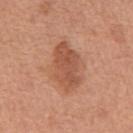Impression:
Imaged during a routine full-body skin examination; the lesion was not biopsied and no histopathology is available.
Acquisition and patient details:
The recorded lesion diameter is about 6 mm. A male patient, roughly 65 years of age. A lesion tile, about 15 mm wide, cut from a 3D total-body photograph. Automated tile analysis of the lesion measured lesion-presence confidence of about 100/100. On the abdomen.